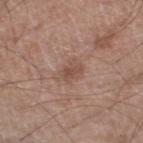Impression: No biopsy was performed on this lesion — it was imaged during a full skin examination and was not determined to be concerning. Context: A region of skin cropped from a whole-body photographic capture, roughly 15 mm wide. An algorithmic analysis of the crop reported an area of roughly 5 mm², an outline eccentricity of about 0.65 (0 = round, 1 = elongated), and two-axis asymmetry of about 0.2. The analysis additionally found about 8 CIELAB-L* units darker than the surrounding skin and a normalized lesion–skin contrast near 6. The analysis additionally found a border-irregularity rating of about 2.5/10, a within-lesion color-variation index near 2.5/10, and radial color variation of about 1. The software also gave an automated nevus-likeness rating near 5 out of 100 and lesion-presence confidence of about 100/100. This is a white-light tile. The recorded lesion diameter is about 3 mm. A male patient aged 58 to 62. From the right lower leg.